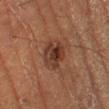– notes · catalogued during a skin exam; not biopsied
– patient · male, aged 63–67
– image · 15 mm crop, total-body photography
– size · ≈3.5 mm
– location · the leg